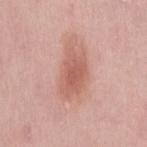workup: total-body-photography surveillance lesion; no biopsy | tile lighting: white-light | body site: the lower back | TBP lesion metrics: a footprint of about 12 mm², an eccentricity of roughly 0.85, and a shape-asymmetry score of about 0.2 (0 = symmetric); an average lesion color of about L≈59 a*≈25 b*≈27 (CIELAB) and roughly 10 lightness units darker than nearby skin; a border-irregularity rating of about 3/10 and peripheral color asymmetry of about 1; a classifier nevus-likeness of about 95/100 and lesion-presence confidence of about 100/100 | imaging modality: 15 mm crop, total-body photography | subject: female, aged around 30 | size: ≈5.5 mm.